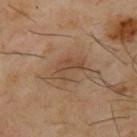biopsy status: catalogued during a skin exam; not biopsied
site: the upper back
image source: total-body-photography crop, ~15 mm field of view
subject: male, roughly 65 years of age
diameter: about 4.5 mm